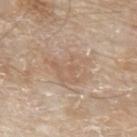Q: Was a biopsy performed?
A: no biopsy performed (imaged during a skin exam)
Q: What kind of image is this?
A: total-body-photography crop, ~15 mm field of view
Q: Where on the body is the lesion?
A: the left forearm
Q: Patient demographics?
A: male, aged approximately 80